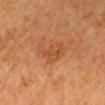Part of a total-body skin-imaging series; this lesion was reviewed on a skin check and was not flagged for biopsy. A region of skin cropped from a whole-body photographic capture, roughly 15 mm wide. The tile uses cross-polarized illumination. A female patient approximately 65 years of age. Located on the head or neck. The lesion-visualizer software estimated a normalized border contrast of about 5.5. It also reported an automated nevus-likeness rating near 5 out of 100 and a lesion-detection confidence of about 100/100.